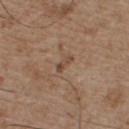Imaged during a routine full-body skin examination; the lesion was not biopsied and no histopathology is available. Approximately 2.5 mm at its widest. The total-body-photography lesion software estimated a lesion area of about 2.5 mm² and two-axis asymmetry of about 0.4. The analysis additionally found border irregularity of about 4 on a 0–10 scale, internal color variation of about 0 on a 0–10 scale, and peripheral color asymmetry of about 0. The analysis additionally found a classifier nevus-likeness of about 0/100. A 15 mm crop from a total-body photograph taken for skin-cancer surveillance. The subject is a male about 55 years old. This is a white-light tile. The lesion is located on the chest.